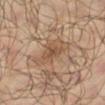Captured during whole-body skin photography for melanoma surveillance; the lesion was not biopsied.
A region of skin cropped from a whole-body photographic capture, roughly 15 mm wide.
A male subject, about 65 years old.
From the leg.